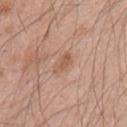Q: Was this lesion biopsied?
A: catalogued during a skin exam; not biopsied
Q: How was this image acquired?
A: ~15 mm tile from a whole-body skin photo
Q: Automated lesion metrics?
A: roughly 8 lightness units darker than nearby skin and a normalized lesion–skin contrast near 6; a border-irregularity rating of about 3/10 and a peripheral color-asymmetry measure near 0; a nevus-likeness score of about 5/100
Q: What are the patient's age and sex?
A: male, approximately 45 years of age
Q: Illumination type?
A: white-light illumination
Q: How large is the lesion?
A: ≈3 mm
Q: Lesion location?
A: the left upper arm